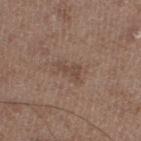This lesion was catalogued during total-body skin photography and was not selected for biopsy.
A 15 mm close-up extracted from a 3D total-body photography capture.
This is a white-light tile.
The lesion's longest dimension is about 3 mm.
On the left lower leg.
The patient is a male aged 48 to 52.